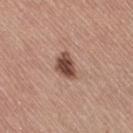biopsy_status: not biopsied; imaged during a skin examination
lighting: white-light
lesion_size:
  long_diameter_mm_approx: 3.5
patient:
  sex: female
  age_approx: 65
site: right thigh
image:
  source: total-body photography crop
  field_of_view_mm: 15
automated_metrics:
  nevus_likeness_0_100: 95
  lesion_detection_confidence_0_100: 100